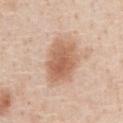notes: no biopsy performed (imaged during a skin exam)
body site: the chest
lighting: white-light illumination
image: 15 mm crop, total-body photography
patient: male, aged 58 to 62
automated lesion analysis: an area of roughly 17 mm², an outline eccentricity of about 0.7 (0 = round, 1 = elongated), and a shape-asymmetry score of about 0.2 (0 = symmetric); a lesion color around L≈62 a*≈21 b*≈32 in CIELAB and roughly 12 lightness units darker than nearby skin; an automated nevus-likeness rating near 95 out of 100 and lesion-presence confidence of about 100/100
lesion size: ≈5.5 mm